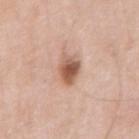Recorded during total-body skin imaging; not selected for excision or biopsy.
The lesion is located on the right upper arm.
A 15 mm crop from a total-body photograph taken for skin-cancer surveillance.
The recorded lesion diameter is about 3.5 mm.
The tile uses white-light illumination.
Automated tile analysis of the lesion measured roughly 15 lightness units darker than nearby skin and a lesion-to-skin contrast of about 10 (normalized; higher = more distinct). And it measured an automated nevus-likeness rating near 95 out of 100 and a lesion-detection confidence of about 100/100.
The subject is a male in their mid-60s.